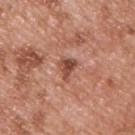Assessment: Captured during whole-body skin photography for melanoma surveillance; the lesion was not biopsied. Context: A male patient about 55 years old. Automated tile analysis of the lesion measured a lesion area of about 4 mm² and a symmetry-axis asymmetry near 0.5. It also reported a border-irregularity rating of about 4.5/10, a color-variation rating of about 5/10, and peripheral color asymmetry of about 2. It also reported a nevus-likeness score of about 0/100 and a detector confidence of about 100 out of 100 that the crop contains a lesion. A region of skin cropped from a whole-body photographic capture, roughly 15 mm wide. The lesion is located on the upper back. Approximately 3 mm at its widest. Captured under white-light illumination.